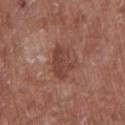Q: Was this lesion biopsied?
A: total-body-photography surveillance lesion; no biopsy
Q: What lighting was used for the tile?
A: white-light illumination
Q: What is the imaging modality?
A: total-body-photography crop, ~15 mm field of view
Q: Automated lesion metrics?
A: a shape-asymmetry score of about 0.2 (0 = symmetric)
Q: Where on the body is the lesion?
A: the front of the torso
Q: What are the patient's age and sex?
A: male, aged 73 to 77
Q: Lesion size?
A: ≈4 mm